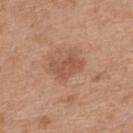The lesion was tiled from a total-body skin photograph and was not biopsied.
The lesion-visualizer software estimated a shape eccentricity near 0.75 and a symmetry-axis asymmetry near 0.3. And it measured an average lesion color of about L≈53 a*≈21 b*≈32 (CIELAB), roughly 8 lightness units darker than nearby skin, and a normalized border contrast of about 6. The software also gave border irregularity of about 4 on a 0–10 scale, a color-variation rating of about 2.5/10, and peripheral color asymmetry of about 1. And it measured an automated nevus-likeness rating near 0 out of 100 and a lesion-detection confidence of about 100/100.
This image is a 15 mm lesion crop taken from a total-body photograph.
Longest diameter approximately 4 mm.
A female subject aged approximately 40.
Captured under white-light illumination.
On the upper back.